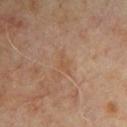Impression:
Imaged during a routine full-body skin examination; the lesion was not biopsied and no histopathology is available.
Background:
The patient is a male aged around 65. From the chest. Approximately 3 mm at its widest. A 15 mm crop from a total-body photograph taken for skin-cancer surveillance. This is a cross-polarized tile.